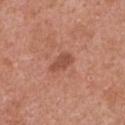Assessment: The lesion was tiled from a total-body skin photograph and was not biopsied. Background: The lesion is on the chest. A female subject aged around 50. The lesion-visualizer software estimated an area of roughly 4 mm², an eccentricity of roughly 0.85, and two-axis asymmetry of about 0.4. It also reported a border-irregularity index near 3.5/10, a within-lesion color-variation index near 1/10, and a peripheral color-asymmetry measure near 0.5. The software also gave an automated nevus-likeness rating near 45 out of 100 and a detector confidence of about 100 out of 100 that the crop contains a lesion. A 15 mm crop from a total-body photograph taken for skin-cancer surveillance. Captured under white-light illumination. Longest diameter approximately 3 mm.